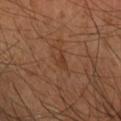Impression:
The lesion was photographed on a routine skin check and not biopsied; there is no pathology result.
Acquisition and patient details:
The tile uses cross-polarized illumination. The lesion is located on the right forearm. A roughly 15 mm field-of-view crop from a total-body skin photograph. The lesion's longest dimension is about 2.5 mm. A male subject, about 50 years old. The total-body-photography lesion software estimated a footprint of about 3.5 mm² and a shape-asymmetry score of about 0.4 (0 = symmetric). The software also gave a lesion color around L≈38 a*≈21 b*≈31 in CIELAB and a normalized border contrast of about 5. And it measured a color-variation rating of about 1.5/10 and peripheral color asymmetry of about 0.5.